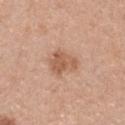No biopsy was performed on this lesion — it was imaged during a full skin examination and was not determined to be concerning. A female patient aged 28 to 32. A 15 mm close-up extracted from a 3D total-body photography capture. The lesion-visualizer software estimated a lesion color around L≈57 a*≈22 b*≈32 in CIELAB, roughly 10 lightness units darker than nearby skin, and a normalized border contrast of about 7. It also reported a border-irregularity index near 3.5/10 and a color-variation rating of about 2.5/10. Approximately 3.5 mm at its widest. On the chest.